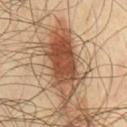Impression: No biopsy was performed on this lesion — it was imaged during a full skin examination and was not determined to be concerning. Context: Located on the chest. The subject is a male aged 63 to 67. A 15 mm crop from a total-body photograph taken for skin-cancer surveillance.